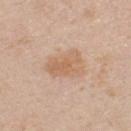notes: total-body-photography surveillance lesion; no biopsy
imaging modality: ~15 mm tile from a whole-body skin photo
anatomic site: the chest
TBP lesion metrics: an area of roughly 11 mm², an eccentricity of roughly 0.75, and a shape-asymmetry score of about 0.25 (0 = symmetric); a mean CIELAB color near L≈63 a*≈18 b*≈33, a lesion–skin lightness drop of about 8, and a lesion-to-skin contrast of about 6 (normalized; higher = more distinct)
diameter: ≈4.5 mm
tile lighting: white-light
patient: male, aged approximately 45